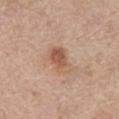Clinical summary:
The recorded lesion diameter is about 4 mm. A lesion tile, about 15 mm wide, cut from a 3D total-body photograph. The tile uses white-light illumination. The total-body-photography lesion software estimated a classifier nevus-likeness of about 30/100 and a detector confidence of about 100 out of 100 that the crop contains a lesion. The patient is a male in their mid-60s. Located on the abdomen.